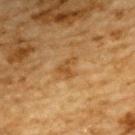follow-up = no biopsy performed (imaged during a skin exam).